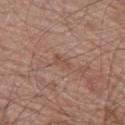<tbp_lesion>
<biopsy_status>not biopsied; imaged during a skin examination</biopsy_status>
<lighting>white-light</lighting>
<patient>
  <sex>male</sex>
  <age_approx>60</age_approx>
</patient>
<image>
  <source>total-body photography crop</source>
  <field_of_view_mm>15</field_of_view_mm>
</image>
<site>left thigh</site>
</tbp_lesion>